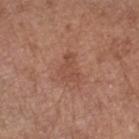Image and clinical context: About 3.5 mm across. The patient is a female aged 63–67. Cropped from a whole-body photographic skin survey; the tile spans about 15 mm. Located on the left forearm. This is a white-light tile. An algorithmic analysis of the crop reported about 6 CIELAB-L* units darker than the surrounding skin and a normalized lesion–skin contrast near 5. And it measured a nevus-likeness score of about 0/100 and a lesion-detection confidence of about 100/100.